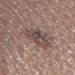Q: Was a biopsy performed?
A: no biopsy performed (imaged during a skin exam)
Q: What are the patient's age and sex?
A: female, approximately 20 years of age
Q: What kind of image is this?
A: ~15 mm crop, total-body skin-cancer survey
Q: Where on the body is the lesion?
A: the leg